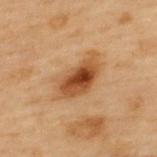| key | value |
|---|---|
| patient | male, aged 53–57 |
| imaging modality | 15 mm crop, total-body photography |
| illumination | cross-polarized illumination |
| site | the back |